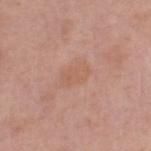No biopsy was performed on this lesion — it was imaged during a full skin examination and was not determined to be concerning. A female subject, roughly 55 years of age. From the leg. A close-up tile cropped from a whole-body skin photograph, about 15 mm across.